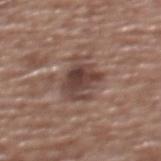follow-up: catalogued during a skin exam; not biopsied
image source: total-body-photography crop, ~15 mm field of view
location: the upper back
tile lighting: white-light illumination
subject: female, in their mid-70s
size: about 4.5 mm